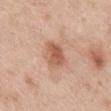Assessment: The lesion was photographed on a routine skin check and not biopsied; there is no pathology result. Background: Cropped from a whole-body photographic skin survey; the tile spans about 15 mm. About 3 mm across. Automated image analysis of the tile measured an outline eccentricity of about 0.6 (0 = round, 1 = elongated) and two-axis asymmetry of about 0.2. And it measured an average lesion color of about L≈58 a*≈22 b*≈31 (CIELAB), roughly 11 lightness units darker than nearby skin, and a normalized lesion–skin contrast near 7.5. The software also gave a lesion-detection confidence of about 100/100. Imaged with white-light lighting. A female subject, aged around 40. From the mid back.